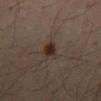Recorded during total-body skin imaging; not selected for excision or biopsy. A region of skin cropped from a whole-body photographic capture, roughly 15 mm wide. Imaged with cross-polarized lighting. Automated tile analysis of the lesion measured a footprint of about 4.5 mm², an outline eccentricity of about 0.8 (0 = round, 1 = elongated), and a shape-asymmetry score of about 0.35 (0 = symmetric). It also reported an average lesion color of about L≈27 a*≈14 b*≈21 (CIELAB), a lesion–skin lightness drop of about 9, and a normalized border contrast of about 10. It also reported a border-irregularity index near 3.5/10 and a peripheral color-asymmetry measure near 1. It also reported an automated nevus-likeness rating near 90 out of 100 and a detector confidence of about 100 out of 100 that the crop contains a lesion. A male subject, roughly 65 years of age. The lesion is located on the front of the torso. Approximately 3 mm at its widest.